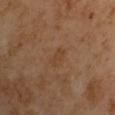notes=catalogued during a skin exam; not biopsied